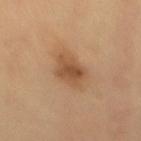| field | value |
|---|---|
| follow-up | no biopsy performed (imaged during a skin exam) |
| patient | male, aged 48–52 |
| location | the lower back |
| imaging modality | 15 mm crop, total-body photography |